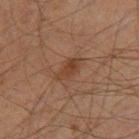biopsy status = imaged on a skin check; not biopsied | patient = male, aged approximately 60 | image = total-body-photography crop, ~15 mm field of view | location = the leg | lighting = cross-polarized.